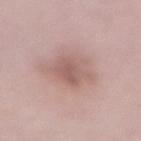workup: no biopsy performed (imaged during a skin exam)
subject: female, aged 48–52
TBP lesion metrics: a lesion color around L≈58 a*≈20 b*≈22 in CIELAB, a lesion–skin lightness drop of about 9, and a normalized border contrast of about 6; a border-irregularity rating of about 3/10, a within-lesion color-variation index near 3.5/10, and radial color variation of about 1.5; a classifier nevus-likeness of about 5/100 and a detector confidence of about 100 out of 100 that the crop contains a lesion
anatomic site: the front of the torso
image source: ~15 mm tile from a whole-body skin photo
illumination: white-light illumination
lesion size: about 4.5 mm